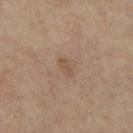Q: Was a biopsy performed?
A: imaged on a skin check; not biopsied
Q: Illumination type?
A: cross-polarized illumination
Q: What is the imaging modality?
A: total-body-photography crop, ~15 mm field of view
Q: What is the lesion's diameter?
A: ≈2.5 mm
Q: Automated lesion metrics?
A: an area of roughly 3.5 mm², a shape eccentricity near 0.75, and a shape-asymmetry score of about 0.25 (0 = symmetric); an average lesion color of about L≈53 a*≈17 b*≈29 (CIELAB) and a lesion–skin lightness drop of about 6
Q: Who is the patient?
A: female, about 65 years old
Q: Where on the body is the lesion?
A: the right lower leg